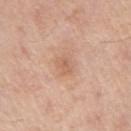The lesion was photographed on a routine skin check and not biopsied; there is no pathology result. The lesion is located on the left upper arm. An algorithmic analysis of the crop reported an area of roughly 3 mm², an eccentricity of roughly 0.8, and two-axis asymmetry of about 0.35. A male subject aged 53 to 57. About 2.5 mm across. A 15 mm close-up extracted from a 3D total-body photography capture.